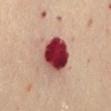Q: Is there a histopathology result?
A: total-body-photography surveillance lesion; no biopsy
Q: Who is the patient?
A: female, aged around 60
Q: How was this image acquired?
A: ~15 mm crop, total-body skin-cancer survey
Q: What is the lesion's diameter?
A: ≈4.5 mm
Q: Lesion location?
A: the abdomen
Q: Illumination type?
A: cross-polarized illumination